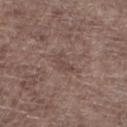Context: A close-up tile cropped from a whole-body skin photograph, about 15 mm across. A male subject, aged 68 to 72. The total-body-photography lesion software estimated roughly 6 lightness units darker than nearby skin and a lesion-to-skin contrast of about 5 (normalized; higher = more distinct). The recorded lesion diameter is about 3 mm. Captured under white-light illumination. On the right lower leg.